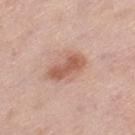notes: total-body-photography surveillance lesion; no biopsy
site: the left thigh
lesion size: about 5 mm
image source: ~15 mm tile from a whole-body skin photo
subject: male, aged 58 to 62